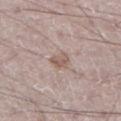Recorded during total-body skin imaging; not selected for excision or biopsy. A male patient approximately 50 years of age. Longest diameter approximately 2.5 mm. A 15 mm close-up tile from a total-body photography series done for melanoma screening. Located on the left lower leg.